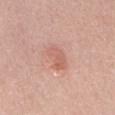Clinical impression:
Imaged during a routine full-body skin examination; the lesion was not biopsied and no histopathology is available.
Background:
Approximately 3.5 mm at its widest. From the abdomen. A male patient, about 50 years old. The tile uses white-light illumination. A 15 mm close-up tile from a total-body photography series done for melanoma screening.